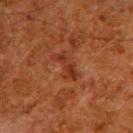The lesion was tiled from a total-body skin photograph and was not biopsied. An algorithmic analysis of the crop reported a mean CIELAB color near L≈27 a*≈23 b*≈28, roughly 6 lightness units darker than nearby skin, and a normalized lesion–skin contrast near 6. It also reported internal color variation of about 2.5 on a 0–10 scale and peripheral color asymmetry of about 0.5. And it measured a detector confidence of about 95 out of 100 that the crop contains a lesion. This is a cross-polarized tile. A lesion tile, about 15 mm wide, cut from a 3D total-body photograph. Measured at roughly 4 mm in maximum diameter. A male subject, approximately 60 years of age. From the upper back.